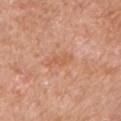{
  "biopsy_status": "not biopsied; imaged during a skin examination",
  "site": "left upper arm",
  "image": {
    "source": "total-body photography crop",
    "field_of_view_mm": 15
  },
  "patient": {
    "sex": "male",
    "age_approx": 60
  },
  "automated_metrics": {
    "area_mm2_approx": 3.5,
    "shape_asymmetry": 0.4,
    "nevus_likeness_0_100": 0,
    "lesion_detection_confidence_0_100": 100
  }
}